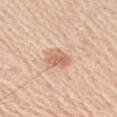<tbp_lesion>
<biopsy_status>not biopsied; imaged during a skin examination</biopsy_status>
<site>right upper arm</site>
<patient>
  <sex>male</sex>
  <age_approx>60</age_approx>
</patient>
<image>
  <source>total-body photography crop</source>
  <field_of_view_mm>15</field_of_view_mm>
</image>
</tbp_lesion>